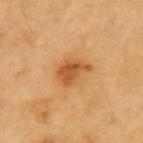Assessment:
Imaged during a routine full-body skin examination; the lesion was not biopsied and no histopathology is available.
Background:
Imaged with cross-polarized lighting. The subject is a male about 60 years old. The lesion's longest dimension is about 4 mm. Cropped from a total-body skin-imaging series; the visible field is about 15 mm. The total-body-photography lesion software estimated a footprint of about 7.5 mm², an outline eccentricity of about 0.75 (0 = round, 1 = elongated), and a symmetry-axis asymmetry near 0.3. The analysis additionally found an average lesion color of about L≈54 a*≈25 b*≈43 (CIELAB), about 11 CIELAB-L* units darker than the surrounding skin, and a lesion-to-skin contrast of about 7.5 (normalized; higher = more distinct). It also reported a classifier nevus-likeness of about 85/100 and lesion-presence confidence of about 100/100. On the left upper arm.